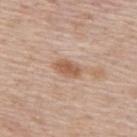The lesion was tiled from a total-body skin photograph and was not biopsied. A close-up tile cropped from a whole-body skin photograph, about 15 mm across. The tile uses white-light illumination. A male subject, aged approximately 75. On the upper back.